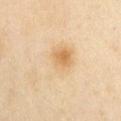This lesion was catalogued during total-body skin photography and was not selected for biopsy.
The lesion is on the chest.
A female patient approximately 45 years of age.
A roughly 15 mm field-of-view crop from a total-body skin photograph.